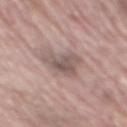Q: Was a biopsy performed?
A: imaged on a skin check; not biopsied
Q: Illumination type?
A: white-light illumination
Q: What are the patient's age and sex?
A: male, about 70 years old
Q: What is the imaging modality?
A: ~15 mm tile from a whole-body skin photo
Q: Automated lesion metrics?
A: a footprint of about 8.5 mm² and an eccentricity of roughly 0.55; a detector confidence of about 90 out of 100 that the crop contains a lesion
Q: Lesion size?
A: ≈4 mm
Q: Where on the body is the lesion?
A: the mid back